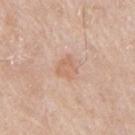A close-up tile cropped from a whole-body skin photograph, about 15 mm across.
Measured at roughly 3 mm in maximum diameter.
Located on the arm.
A male patient, aged around 65.